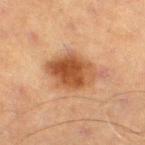- follow-up — catalogued during a skin exam; not biopsied
- image — total-body-photography crop, ~15 mm field of view
- subject — male, aged 68–72
- tile lighting — cross-polarized
- lesion diameter — ~5.5 mm (longest diameter)
- TBP lesion metrics — a footprint of about 16 mm² and a shape-asymmetry score of about 0.15 (0 = symmetric); a mean CIELAB color near L≈43 a*≈21 b*≈32, about 12 CIELAB-L* units darker than the surrounding skin, and a lesion-to-skin contrast of about 9.5 (normalized; higher = more distinct); an automated nevus-likeness rating near 90 out of 100 and lesion-presence confidence of about 100/100
- anatomic site — the right thigh